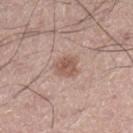Assessment:
No biopsy was performed on this lesion — it was imaged during a full skin examination and was not determined to be concerning.
Background:
This image is a 15 mm lesion crop taken from a total-body photograph. Imaged with white-light lighting. A male subject, aged 48 to 52. Approximately 3 mm at its widest. Located on the leg.